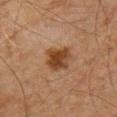Impression:
The lesion was photographed on a routine skin check and not biopsied; there is no pathology result.
Clinical summary:
A close-up tile cropped from a whole-body skin photograph, about 15 mm across. About 3.5 mm across. The lesion is on the chest. This is a cross-polarized tile. A male patient aged 58 to 62.